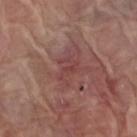No biopsy was performed on this lesion — it was imaged during a full skin examination and was not determined to be concerning. The total-body-photography lesion software estimated a normalized border contrast of about 5. This image is a 15 mm lesion crop taken from a total-body photograph. Located on the right forearm. Imaged with white-light lighting. A male subject, roughly 80 years of age. About 3.5 mm across.